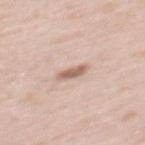biopsy status: total-body-photography surveillance lesion; no biopsy | patient: female, roughly 60 years of age | lesion diameter: about 2.5 mm | image source: ~15 mm tile from a whole-body skin photo | site: the mid back | tile lighting: white-light | image-analysis metrics: a lesion color around L≈61 a*≈19 b*≈27 in CIELAB, roughly 13 lightness units darker than nearby skin, and a normalized lesion–skin contrast near 8.5.